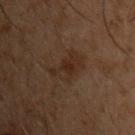| feature | finding |
|---|---|
| biopsy status | no biopsy performed (imaged during a skin exam) |
| patient | male, approximately 50 years of age |
| site | the chest |
| tile lighting | cross-polarized |
| diameter | ≈4.5 mm |
| image source | ~15 mm tile from a whole-body skin photo |